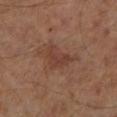workup: no biopsy performed (imaged during a skin exam)
automated metrics: a footprint of about 6 mm², an outline eccentricity of about 0.65 (0 = round, 1 = elongated), and a symmetry-axis asymmetry near 0.5; an average lesion color of about L≈37 a*≈21 b*≈25 (CIELAB) and a normalized border contrast of about 5.5; a border-irregularity index near 5/10, a within-lesion color-variation index near 1.5/10, and peripheral color asymmetry of about 0.5; lesion-presence confidence of about 100/100
lighting: cross-polarized
subject: male, aged 63–67
diameter: ≈3.5 mm
image source: ~15 mm crop, total-body skin-cancer survey